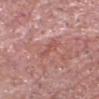Captured under white-light illumination. On the head or neck. A male subject, approximately 65 years of age. The lesion's longest dimension is about 3 mm. A 15 mm close-up tile from a total-body photography series done for melanoma screening.